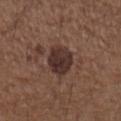Clinical impression: Recorded during total-body skin imaging; not selected for excision or biopsy. Acquisition and patient details: The subject is a male aged around 50. The lesion is on the left upper arm. The tile uses white-light illumination. Cropped from a total-body skin-imaging series; the visible field is about 15 mm.